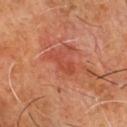{
  "biopsy_status": "not biopsied; imaged during a skin examination",
  "automated_metrics": {
    "cielab_L": 44,
    "cielab_a": 30,
    "cielab_b": 32,
    "vs_skin_contrast_norm": 5.0,
    "nevus_likeness_0_100": 0,
    "lesion_detection_confidence_0_100": 100
  },
  "site": "chest",
  "image": {
    "source": "total-body photography crop",
    "field_of_view_mm": 15
  },
  "patient": {
    "sex": "male",
    "age_approx": 60
  }
}